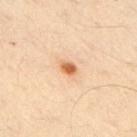Impression: Imaged during a routine full-body skin examination; the lesion was not biopsied and no histopathology is available. Context: Cropped from a whole-body photographic skin survey; the tile spans about 15 mm. A male patient, about 45 years old. The lesion's longest dimension is about 2 mm. The lesion is located on the right upper arm. Automated image analysis of the tile measured an area of roughly 2.5 mm² and a shape eccentricity near 0.7. The software also gave border irregularity of about 2.5 on a 0–10 scale, a color-variation rating of about 2.5/10, and radial color variation of about 0.5. The analysis additionally found lesion-presence confidence of about 100/100.